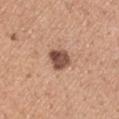Notes:
* follow-up · catalogued during a skin exam; not biopsied
* acquisition · 15 mm crop, total-body photography
* subject · male, aged 43 to 47
* anatomic site · the right upper arm